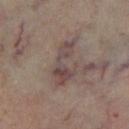Case summary:
* subject: male, aged around 70
* illumination: cross-polarized illumination
* acquisition: total-body-photography crop, ~15 mm field of view
* body site: the leg